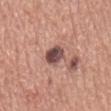Impression: Imaged during a routine full-body skin examination; the lesion was not biopsied and no histopathology is available. Image and clinical context: Imaged with white-light lighting. Automated image analysis of the tile measured a lesion area of about 5.5 mm², an eccentricity of roughly 0.55, and a symmetry-axis asymmetry near 0.1. And it measured a detector confidence of about 100 out of 100 that the crop contains a lesion. The lesion's longest dimension is about 3 mm. From the mid back. The subject is a male in their mid-70s. A close-up tile cropped from a whole-body skin photograph, about 15 mm across.